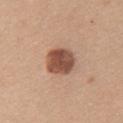The lesion was tiled from a total-body skin photograph and was not biopsied. A female subject, aged 33 to 37. Cropped from a whole-body photographic skin survey; the tile spans about 15 mm. The lesion is located on the left upper arm.